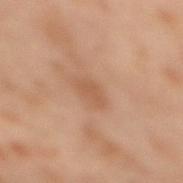No biopsy was performed on this lesion — it was imaged during a full skin examination and was not determined to be concerning.